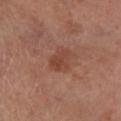Q: How was this image acquired?
A: ~15 mm crop, total-body skin-cancer survey
Q: What lighting was used for the tile?
A: cross-polarized
Q: Where on the body is the lesion?
A: the left lower leg
Q: What did automated image analysis measure?
A: a lesion color around L≈42 a*≈23 b*≈28 in CIELAB and a normalized lesion–skin contrast near 6; a border-irregularity index near 2.5/10, a within-lesion color-variation index near 2.5/10, and peripheral color asymmetry of about 1; a nevus-likeness score of about 15/100 and lesion-presence confidence of about 100/100
Q: What are the patient's age and sex?
A: male, aged 63 to 67